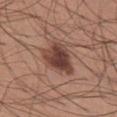Case summary:
• follow-up — imaged on a skin check; not biopsied
• site — the chest
• TBP lesion metrics — a classifier nevus-likeness of about 80/100
• illumination — white-light illumination
• acquisition — ~15 mm tile from a whole-body skin photo
• subject — male, aged approximately 30
• lesion diameter — about 5 mm